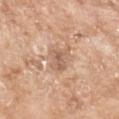biopsy status: catalogued during a skin exam; not biopsied | diameter: ~3.5 mm (longest diameter) | anatomic site: the left forearm | lighting: white-light illumination | image-analysis metrics: an eccentricity of roughly 0.8 and a symmetry-axis asymmetry near 0.35; a mean CIELAB color near L≈60 a*≈19 b*≈31, roughly 10 lightness units darker than nearby skin, and a normalized lesion–skin contrast near 6; a border-irregularity index near 3.5/10, a within-lesion color-variation index near 3.5/10, and peripheral color asymmetry of about 1.5; an automated nevus-likeness rating near 0 out of 100 | acquisition: total-body-photography crop, ~15 mm field of view | subject: female, aged around 75.